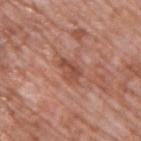No biopsy was performed on this lesion — it was imaged during a full skin examination and was not determined to be concerning.
Imaged with white-light lighting.
A male subject, aged approximately 70.
Cropped from a whole-body photographic skin survey; the tile spans about 15 mm.
The lesion's longest dimension is about 3 mm.
Automated image analysis of the tile measured an eccentricity of roughly 0.8 and a shape-asymmetry score of about 0.3 (0 = symmetric). The software also gave a mean CIELAB color near L≈49 a*≈25 b*≈29, roughly 9 lightness units darker than nearby skin, and a normalized border contrast of about 6.5. And it measured a border-irregularity index near 3.5/10 and a peripheral color-asymmetry measure near 1. The analysis additionally found lesion-presence confidence of about 100/100.
From the upper back.